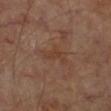Q: Was this lesion biopsied?
A: no biopsy performed (imaged during a skin exam)